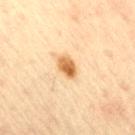Findings:
– biopsy status · no biopsy performed (imaged during a skin exam)
– image source · ~15 mm crop, total-body skin-cancer survey
– tile lighting · cross-polarized illumination
– image-analysis metrics · an area of roughly 4.5 mm², an eccentricity of roughly 0.75, and a symmetry-axis asymmetry near 0.2; an average lesion color of about L≈60 a*≈20 b*≈41 (CIELAB), about 14 CIELAB-L* units darker than the surrounding skin, and a normalized border contrast of about 10; a border-irregularity index near 2/10 and radial color variation of about 2; lesion-presence confidence of about 100/100
– size · ≈3 mm
– anatomic site · the right thigh
– subject · male, about 65 years old